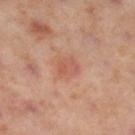The lesion was photographed on a routine skin check and not biopsied; there is no pathology result. The total-body-photography lesion software estimated an area of roughly 4.5 mm², an eccentricity of roughly 0.55, and a symmetry-axis asymmetry near 0.2. The recorded lesion diameter is about 2.5 mm. Located on the right lower leg. Captured under cross-polarized illumination. The subject is a female aged 53–57. A region of skin cropped from a whole-body photographic capture, roughly 15 mm wide.